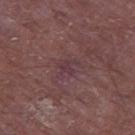Assessment: Captured during whole-body skin photography for melanoma surveillance; the lesion was not biopsied. Image and clinical context: An algorithmic analysis of the crop reported a lesion area of about 3.5 mm². And it measured a border-irregularity index near 6.5/10 and a peripheral color-asymmetry measure near 0.5. This image is a 15 mm lesion crop taken from a total-body photograph. A male subject, aged approximately 65. Measured at roughly 2.5 mm in maximum diameter. From the left thigh.